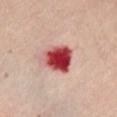Q: Was a biopsy performed?
A: no biopsy performed (imaged during a skin exam)
Q: Where on the body is the lesion?
A: the chest
Q: How large is the lesion?
A: ~4.5 mm (longest diameter)
Q: What did automated image analysis measure?
A: a border-irregularity index near 2.5/10 and a within-lesion color-variation index near 9/10
Q: How was this image acquired?
A: ~15 mm crop, total-body skin-cancer survey
Q: Patient demographics?
A: female, aged around 60